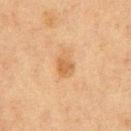- biopsy status: no biopsy performed (imaged during a skin exam)
- imaging modality: ~15 mm crop, total-body skin-cancer survey
- body site: the chest
- lighting: cross-polarized illumination
- patient: male, aged 73 to 77
- diameter: ~3.5 mm (longest diameter)